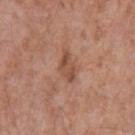Case summary:
- automated lesion analysis: an outline eccentricity of about 0.8 (0 = round, 1 = elongated); a border-irregularity rating of about 3.5/10, a within-lesion color-variation index near 3.5/10, and a peripheral color-asymmetry measure near 1; a nevus-likeness score of about 0/100 and lesion-presence confidence of about 100/100
- body site: the left upper arm
- image source: 15 mm crop, total-body photography
- patient: male, aged 53 to 57